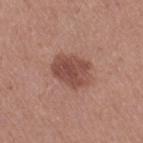Q: Was a biopsy performed?
A: no biopsy performed (imaged during a skin exam)
Q: What is the lesion's diameter?
A: about 4.5 mm
Q: What are the patient's age and sex?
A: female, roughly 40 years of age
Q: Illumination type?
A: white-light
Q: How was this image acquired?
A: total-body-photography crop, ~15 mm field of view
Q: What did automated image analysis measure?
A: a lesion area of about 12 mm² and a symmetry-axis asymmetry near 0.2; a border-irregularity rating of about 2/10, a color-variation rating of about 3.5/10, and radial color variation of about 1.5; a classifier nevus-likeness of about 60/100 and a detector confidence of about 100 out of 100 that the crop contains a lesion
Q: Lesion location?
A: the leg